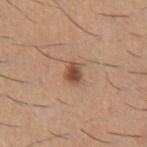Q: Is there a histopathology result?
A: imaged on a skin check; not biopsied
Q: Patient demographics?
A: male, aged 58–62
Q: Lesion location?
A: the left upper arm
Q: What is the lesion's diameter?
A: ≈2.5 mm
Q: What kind of image is this?
A: total-body-photography crop, ~15 mm field of view
Q: What did automated image analysis measure?
A: an area of roughly 4 mm²; an automated nevus-likeness rating near 95 out of 100 and a detector confidence of about 100 out of 100 that the crop contains a lesion